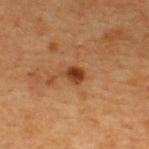Captured during whole-body skin photography for melanoma surveillance; the lesion was not biopsied. The patient is a female aged around 40. The lesion's longest dimension is about 2.5 mm. From the upper back. Captured under cross-polarized illumination. A lesion tile, about 15 mm wide, cut from a 3D total-body photograph.